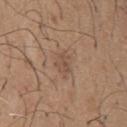follow-up = total-body-photography surveillance lesion; no biopsy
image source = 15 mm crop, total-body photography
site = the chest
patient = male, aged 63–67